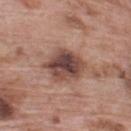No biopsy was performed on this lesion — it was imaged during a full skin examination and was not determined to be concerning.
Cropped from a total-body skin-imaging series; the visible field is about 15 mm.
The lesion is located on the upper back.
A male patient aged 68–72.